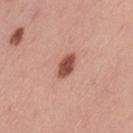Impression:
Captured during whole-body skin photography for melanoma surveillance; the lesion was not biopsied.
Context:
A female patient in their mid-30s. From the leg. A 15 mm close-up tile from a total-body photography series done for melanoma screening.